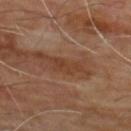- diameter — ≈4 mm
- subject — male, in their mid-60s
- body site — the back
- image source — 15 mm crop, total-body photography